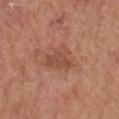Notes:
– workup: total-body-photography surveillance lesion; no biopsy
– TBP lesion metrics: a mean CIELAB color near L≈49 a*≈24 b*≈29, a lesion–skin lightness drop of about 8, and a lesion-to-skin contrast of about 6 (normalized; higher = more distinct); an automated nevus-likeness rating near 0 out of 100
– site: the head or neck
– lesion size: ≈4.5 mm
– imaging modality: ~15 mm tile from a whole-body skin photo
– patient: male, about 55 years old